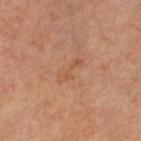– biopsy status: catalogued during a skin exam; not biopsied
– subject: female, in their mid-60s
– acquisition: total-body-photography crop, ~15 mm field of view
– anatomic site: the left lower leg
– automated lesion analysis: a shape eccentricity near 0.95 and two-axis asymmetry of about 0.5; a lesion color around L≈52 a*≈23 b*≈33 in CIELAB, a lesion–skin lightness drop of about 6, and a normalized lesion–skin contrast near 4.5
– size: ~3.5 mm (longest diameter)